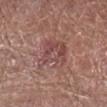Notes:
• follow-up — imaged on a skin check; not biopsied
• lighting — white-light
• image-analysis metrics — a footprint of about 13 mm², an outline eccentricity of about 0.55 (0 = round, 1 = elongated), and two-axis asymmetry of about 0.35; an average lesion color of about L≈47 a*≈23 b*≈21 (CIELAB), roughly 7 lightness units darker than nearby skin, and a normalized border contrast of about 6; a nevus-likeness score of about 0/100 and lesion-presence confidence of about 95/100
• body site — the right lower leg
• lesion size — ≈4.5 mm
• image — 15 mm crop, total-body photography
• patient — male, aged approximately 65